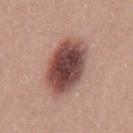Findings:
– notes: no biopsy performed (imaged during a skin exam)
– subject: male, approximately 25 years of age
– anatomic site: the mid back
– acquisition: total-body-photography crop, ~15 mm field of view
– lesion diameter: ≈6.5 mm